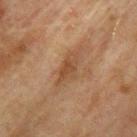Q: Was a biopsy performed?
A: catalogued during a skin exam; not biopsied
Q: What kind of image is this?
A: 15 mm crop, total-body photography
Q: Patient demographics?
A: male, aged 73 to 77
Q: What is the lesion's diameter?
A: ≈4 mm
Q: What lighting was used for the tile?
A: cross-polarized illumination
Q: Where on the body is the lesion?
A: the left upper arm